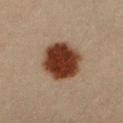Case summary:
• follow-up: total-body-photography surveillance lesion; no biopsy
• tile lighting: cross-polarized illumination
• image-analysis metrics: a footprint of about 18 mm², an eccentricity of roughly 0.45, and a symmetry-axis asymmetry near 0.15
• patient: male, in their mid-30s
• diameter: about 5 mm
• body site: the right forearm
• acquisition: ~15 mm tile from a whole-body skin photo